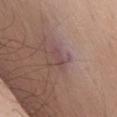Assessment: Recorded during total-body skin imaging; not selected for excision or biopsy. Clinical summary: The patient is a male roughly 70 years of age. A 15 mm crop from a total-body photograph taken for skin-cancer surveillance. The lesion is located on the mid back. Measured at roughly 2.5 mm in maximum diameter.